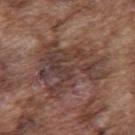Part of a total-body skin-imaging series; this lesion was reviewed on a skin check and was not flagged for biopsy. Cropped from a whole-body photographic skin survey; the tile spans about 15 mm. The subject is a male aged around 75. Located on the upper back. About 8.5 mm across.